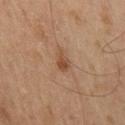Impression: The lesion was tiled from a total-body skin photograph and was not biopsied. Context: A male subject approximately 60 years of age. An algorithmic analysis of the crop reported an area of roughly 3.5 mm², an eccentricity of roughly 0.8, and two-axis asymmetry of about 0.35. And it measured a border-irregularity rating of about 3.5/10, a color-variation rating of about 1.5/10, and peripheral color asymmetry of about 0.5. Captured under cross-polarized illumination. The lesion is located on the leg. A roughly 15 mm field-of-view crop from a total-body skin photograph.